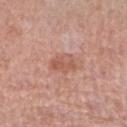This lesion was catalogued during total-body skin photography and was not selected for biopsy.
A female patient, aged around 70.
Cropped from a whole-body photographic skin survey; the tile spans about 15 mm.
This is a white-light tile.
About 3.5 mm across.
From the right lower leg.